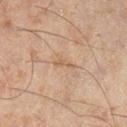Assessment: No biopsy was performed on this lesion — it was imaged during a full skin examination and was not determined to be concerning. Image and clinical context: The lesion-visualizer software estimated a shape eccentricity near 0.95 and a symmetry-axis asymmetry near 0.35. The software also gave an average lesion color of about L≈47 a*≈14 b*≈27 (CIELAB) and about 5 CIELAB-L* units darker than the surrounding skin. It also reported a border-irregularity rating of about 4/10, internal color variation of about 0 on a 0–10 scale, and a peripheral color-asymmetry measure near 0. The software also gave a nevus-likeness score of about 0/100 and a detector confidence of about 95 out of 100 that the crop contains a lesion. Captured under cross-polarized illumination. A male patient aged around 45. A 15 mm close-up extracted from a 3D total-body photography capture. From the right lower leg.